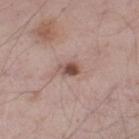Clinical impression: Recorded during total-body skin imaging; not selected for excision or biopsy. Context: From the left thigh. Cropped from a total-body skin-imaging series; the visible field is about 15 mm. A male patient, in their mid-50s. Imaged with white-light lighting.